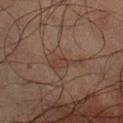follow-up: catalogued during a skin exam; not biopsied
site: the upper back
imaging modality: total-body-photography crop, ~15 mm field of view
subject: male, aged 63–67